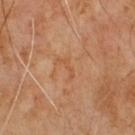This lesion was catalogued during total-body skin photography and was not selected for biopsy. Cropped from a whole-body photographic skin survey; the tile spans about 15 mm. Automated tile analysis of the lesion measured about 5 CIELAB-L* units darker than the surrounding skin and a normalized lesion–skin contrast near 4.5. The patient is a male about 65 years old. This is a cross-polarized tile. Measured at roughly 3 mm in maximum diameter.